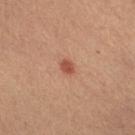No biopsy was performed on this lesion — it was imaged during a full skin examination and was not determined to be concerning.
Cropped from a total-body skin-imaging series; the visible field is about 15 mm.
A female patient, aged around 35.
Captured under cross-polarized illumination.
The lesion is on the right lower leg.
Approximately 2 mm at its widest.